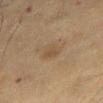follow-up: total-body-photography surveillance lesion; no biopsy